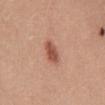workup = no biopsy performed (imaged during a skin exam) | acquisition = ~15 mm crop, total-body skin-cancer survey | tile lighting = white-light | patient = female, in their mid-30s | automated metrics = about 13 CIELAB-L* units darker than the surrounding skin and a normalized border contrast of about 8.5; a border-irregularity rating of about 2/10, internal color variation of about 1.5 on a 0–10 scale, and peripheral color asymmetry of about 0.5; an automated nevus-likeness rating near 95 out of 100 and a lesion-detection confidence of about 100/100 | site = the mid back.